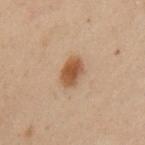| feature | finding |
|---|---|
| follow-up | total-body-photography surveillance lesion; no biopsy |
| size | about 3 mm |
| patient | male, aged 53 to 57 |
| acquisition | total-body-photography crop, ~15 mm field of view |
| TBP lesion metrics | a lesion–skin lightness drop of about 10 and a normalized lesion–skin contrast near 9 |
| illumination | cross-polarized illumination |
| anatomic site | the arm |